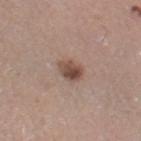Assessment:
The lesion was tiled from a total-body skin photograph and was not biopsied.
Context:
The subject is a female aged approximately 40. From the right thigh. A roughly 15 mm field-of-view crop from a total-body skin photograph.